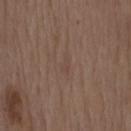workup = no biopsy performed (imaged during a skin exam).